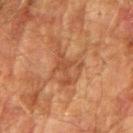Clinical impression: The lesion was tiled from a total-body skin photograph and was not biopsied. Acquisition and patient details: The lesion-visualizer software estimated about 6 CIELAB-L* units darker than the surrounding skin and a normalized border contrast of about 5.5. A close-up tile cropped from a whole-body skin photograph, about 15 mm across. This is a cross-polarized tile. The lesion is located on the right upper arm. Longest diameter approximately 6 mm. A male subject, aged approximately 75.